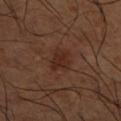<case>
  <biopsy_status>not biopsied; imaged during a skin examination</biopsy_status>
  <patient>
    <sex>male</sex>
    <age_approx>65</age_approx>
  </patient>
  <site>right lower leg</site>
  <automated_metrics>
    <vs_skin_darker_L>7.0</vs_skin_darker_L>
    <vs_skin_contrast_norm>7.5</vs_skin_contrast_norm>
    <border_irregularity_0_10>3.5</border_irregularity_0_10>
    <color_variation_0_10>1.5</color_variation_0_10>
    <peripheral_color_asymmetry>0.5</peripheral_color_asymmetry>
  </automated_metrics>
  <lesion_size>
    <long_diameter_mm_approx>2.5</long_diameter_mm_approx>
  </lesion_size>
  <lighting>cross-polarized</lighting>
  <image>
    <source>total-body photography crop</source>
    <field_of_view_mm>15</field_of_view_mm>
  </image>
</case>